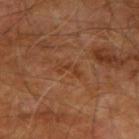{
  "biopsy_status": "not biopsied; imaged during a skin examination",
  "lighting": "cross-polarized",
  "lesion_size": {
    "long_diameter_mm_approx": 3.0
  },
  "image": {
    "source": "total-body photography crop",
    "field_of_view_mm": 15
  },
  "site": "right upper arm",
  "patient": {
    "sex": "male",
    "age_approx": 70
  }
}